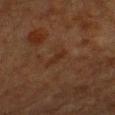Impression:
The lesion was photographed on a routine skin check and not biopsied; there is no pathology result.
Acquisition and patient details:
Automated tile analysis of the lesion measured a border-irregularity rating of about 2.5/10 and radial color variation of about 0. It also reported a classifier nevus-likeness of about 0/100 and lesion-presence confidence of about 100/100. A roughly 15 mm field-of-view crop from a total-body skin photograph. The patient is a female approximately 55 years of age. The lesion is on the head or neck.